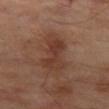Q: Is there a histopathology result?
A: no biopsy performed (imaged during a skin exam)
Q: What kind of image is this?
A: 15 mm crop, total-body photography
Q: Patient demographics?
A: male, aged 68–72
Q: Illumination type?
A: cross-polarized
Q: Where on the body is the lesion?
A: the left thigh
Q: What is the lesion's diameter?
A: ≈6 mm